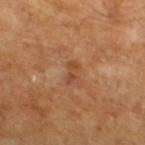Clinical impression:
No biopsy was performed on this lesion — it was imaged during a full skin examination and was not determined to be concerning.
Context:
A male subject, roughly 65 years of age. A 15 mm close-up tile from a total-body photography series done for melanoma screening. Measured at roughly 2.5 mm in maximum diameter. This is a cross-polarized tile. Automated image analysis of the tile measured a lesion color around L≈45 a*≈22 b*≈34 in CIELAB, a lesion–skin lightness drop of about 7, and a lesion-to-skin contrast of about 5.5 (normalized; higher = more distinct). It also reported a lesion-detection confidence of about 100/100.